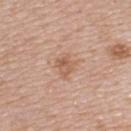On the upper back.
A region of skin cropped from a whole-body photographic capture, roughly 15 mm wide.
A female subject about 45 years old.